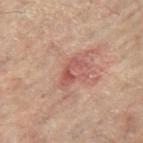workup: catalogued during a skin exam; not biopsied | lesion size: ≈3.5 mm | imaging modality: 15 mm crop, total-body photography | site: the left leg | patient: female, in their 80s | lighting: cross-polarized illumination.